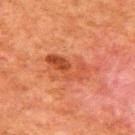{"biopsy_status": "not biopsied; imaged during a skin examination", "site": "upper back", "patient": {"sex": "male", "age_approx": 80}, "image": {"source": "total-body photography crop", "field_of_view_mm": 15}}